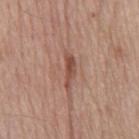Assessment:
Recorded during total-body skin imaging; not selected for excision or biopsy.
Clinical summary:
This image is a 15 mm lesion crop taken from a total-body photograph. The lesion-visualizer software estimated an area of roughly 3.5 mm², an outline eccentricity of about 0.95 (0 = round, 1 = elongated), and a symmetry-axis asymmetry near 0.4. It also reported a lesion color around L≈49 a*≈22 b*≈27 in CIELAB and a lesion-to-skin contrast of about 8 (normalized; higher = more distinct). It also reported an automated nevus-likeness rating near 50 out of 100 and a detector confidence of about 100 out of 100 that the crop contains a lesion. Imaged with white-light lighting. The lesion is on the front of the torso. The subject is a male in their mid- to late 60s.